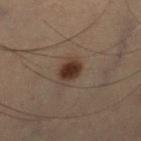This lesion was catalogued during total-body skin photography and was not selected for biopsy.
About 2.5 mm across.
The lesion is located on the right lower leg.
The subject is a male aged 53 to 57.
Imaged with cross-polarized lighting.
Cropped from a whole-body photographic skin survey; the tile spans about 15 mm.
Automated image analysis of the tile measured an average lesion color of about L≈25 a*≈14 b*≈20 (CIELAB), about 11 CIELAB-L* units darker than the surrounding skin, and a normalized border contrast of about 12. It also reported a border-irregularity rating of about 2/10, a color-variation rating of about 2/10, and radial color variation of about 0.5. The analysis additionally found a nevus-likeness score of about 100/100 and lesion-presence confidence of about 100/100.